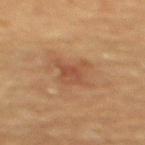The lesion was tiled from a total-body skin photograph and was not biopsied. Captured under cross-polarized illumination. A 15 mm close-up extracted from a 3D total-body photography capture. Longest diameter approximately 3.5 mm. The lesion-visualizer software estimated a lesion area of about 8 mm², a shape eccentricity near 0.5, and two-axis asymmetry of about 0.3. It also reported a nevus-likeness score of about 0/100. A female subject in their 70s. Located on the upper back.